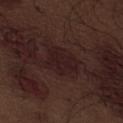Captured during whole-body skin photography for melanoma surveillance; the lesion was not biopsied. The lesion is located on the abdomen. An algorithmic analysis of the crop reported a mean CIELAB color near L≈18 a*≈17 b*≈15. It also reported border irregularity of about 3 on a 0–10 scale and radial color variation of about 0.5. The lesion's longest dimension is about 4.5 mm. A male subject, aged approximately 70. A close-up tile cropped from a whole-body skin photograph, about 15 mm across.